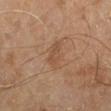The lesion was photographed on a routine skin check and not biopsied; there is no pathology result. Imaged with cross-polarized lighting. The lesion-visualizer software estimated a lesion area of about 7 mm², an outline eccentricity of about 0.85 (0 = round, 1 = elongated), and two-axis asymmetry of about 0.25. The software also gave a border-irregularity rating of about 3.5/10, a color-variation rating of about 2/10, and peripheral color asymmetry of about 1. A close-up tile cropped from a whole-body skin photograph, about 15 mm across. The lesion is on the abdomen. The patient is a male about 60 years old.